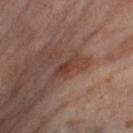The lesion was photographed on a routine skin check and not biopsied; there is no pathology result. A female subject in their mid- to late 50s. The lesion's longest dimension is about 3.5 mm. On the leg. A roughly 15 mm field-of-view crop from a total-body skin photograph.